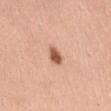biopsy_status: not biopsied; imaged during a skin examination
patient:
  sex: female
  age_approx: 55
image:
  source: total-body photography crop
  field_of_view_mm: 15
lesion_size:
  long_diameter_mm_approx: 2.5
site: abdomen
lighting: white-light